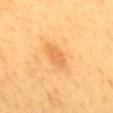workup — imaged on a skin check; not biopsied
lighting — cross-polarized
body site — the back
size — about 3 mm
patient — male, roughly 60 years of age
imaging modality — total-body-photography crop, ~15 mm field of view